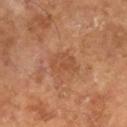This lesion was catalogued during total-body skin photography and was not selected for biopsy.
Imaged with cross-polarized lighting.
A 15 mm close-up tile from a total-body photography series done for melanoma screening.
Measured at roughly 3.5 mm in maximum diameter.
A male subject about 65 years old.